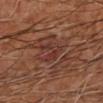Impression:
Part of a total-body skin-imaging series; this lesion was reviewed on a skin check and was not flagged for biopsy.
Image and clinical context:
Longest diameter approximately 3.5 mm. The tile uses cross-polarized illumination. The subject is in their mid- to late 60s. A lesion tile, about 15 mm wide, cut from a 3D total-body photograph. An algorithmic analysis of the crop reported an outline eccentricity of about 0.75 (0 = round, 1 = elongated) and two-axis asymmetry of about 0.6. And it measured border irregularity of about 7.5 on a 0–10 scale and a within-lesion color-variation index near 3/10. It also reported an automated nevus-likeness rating near 0 out of 100 and lesion-presence confidence of about 50/100. The lesion is on the right forearm.